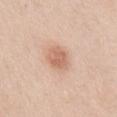Findings:
* follow-up · total-body-photography surveillance lesion; no biopsy
* image · ~15 mm tile from a whole-body skin photo
* patient · female, aged around 60
* lesion size · ~3 mm (longest diameter)
* site · the mid back
* TBP lesion metrics · a lesion area of about 7 mm² and an eccentricity of roughly 0.45; internal color variation of about 3 on a 0–10 scale
* tile lighting · white-light illumination